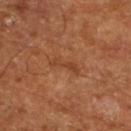The lesion was photographed on a routine skin check and not biopsied; there is no pathology result.
The subject is in their mid- to late 60s.
The lesion is located on the left lower leg.
The recorded lesion diameter is about 4 mm.
Cropped from a total-body skin-imaging series; the visible field is about 15 mm.
Automated tile analysis of the lesion measured a border-irregularity index near 4.5/10 and radial color variation of about 0. It also reported a nevus-likeness score of about 0/100 and lesion-presence confidence of about 100/100.